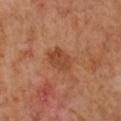Clinical impression: The lesion was photographed on a routine skin check and not biopsied; there is no pathology result. Acquisition and patient details: The lesion's longest dimension is about 3.5 mm. A close-up tile cropped from a whole-body skin photograph, about 15 mm across. The tile uses cross-polarized illumination. A female subject, aged 48 to 52. From the chest.